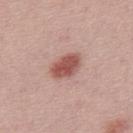Case summary:
• subject: male, aged 28–32
• illumination: white-light illumination
• acquisition: ~15 mm tile from a whole-body skin photo
• lesion size: ~4 mm (longest diameter)
• automated metrics: a lesion area of about 8.5 mm², a shape eccentricity near 0.8, and a shape-asymmetry score of about 0.15 (0 = symmetric); a lesion color around L≈54 a*≈25 b*≈24 in CIELAB, about 13 CIELAB-L* units darker than the surrounding skin, and a normalized border contrast of about 9; a border-irregularity index near 1.5/10, internal color variation of about 3 on a 0–10 scale, and a peripheral color-asymmetry measure near 1